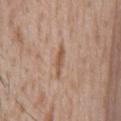No biopsy was performed on this lesion — it was imaged during a full skin examination and was not determined to be concerning. On the chest. A male patient in their mid-40s. A region of skin cropped from a whole-body photographic capture, roughly 15 mm wide.